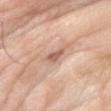Findings:
– biopsy status: imaged on a skin check; not biopsied
– patient: male, about 60 years old
– body site: the left forearm
– acquisition: ~15 mm tile from a whole-body skin photo